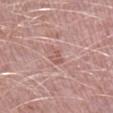Q: Is there a histopathology result?
A: no biopsy performed (imaged during a skin exam)
Q: Patient demographics?
A: male, approximately 50 years of age
Q: What is the lesion's diameter?
A: ≈3 mm
Q: What is the imaging modality?
A: ~15 mm crop, total-body skin-cancer survey
Q: What is the anatomic site?
A: the right lower leg
Q: Illumination type?
A: white-light illumination
Q: Automated lesion metrics?
A: a footprint of about 3 mm², an eccentricity of roughly 0.9, and a symmetry-axis asymmetry near 0.4; border irregularity of about 4.5 on a 0–10 scale, a color-variation rating of about 0.5/10, and radial color variation of about 0; an automated nevus-likeness rating near 0 out of 100 and lesion-presence confidence of about 95/100